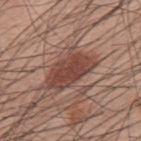Part of a total-body skin-imaging series; this lesion was reviewed on a skin check and was not flagged for biopsy. The tile uses white-light illumination. A close-up tile cropped from a whole-body skin photograph, about 15 mm across. An algorithmic analysis of the crop reported an average lesion color of about L≈44 a*≈22 b*≈25 (CIELAB) and a lesion–skin lightness drop of about 12. The software also gave internal color variation of about 3 on a 0–10 scale and peripheral color asymmetry of about 1. The software also gave a nevus-likeness score of about 85/100 and lesion-presence confidence of about 100/100. The lesion is located on the back. The lesion's longest dimension is about 6 mm. The patient is a male about 60 years old.